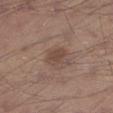Assessment:
Part of a total-body skin-imaging series; this lesion was reviewed on a skin check and was not flagged for biopsy.